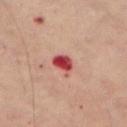No biopsy was performed on this lesion — it was imaged during a full skin examination and was not determined to be concerning. This image is a 15 mm lesion crop taken from a total-body photograph. The lesion-visualizer software estimated a footprint of about 4.5 mm², an outline eccentricity of about 0.7 (0 = round, 1 = elongated), and a shape-asymmetry score of about 0.3 (0 = symmetric). It also reported a normalized border contrast of about 12. The software also gave border irregularity of about 3 on a 0–10 scale, internal color variation of about 4 on a 0–10 scale, and a peripheral color-asymmetry measure near 1.5. A female patient, roughly 60 years of age. This is a cross-polarized tile. The lesion is on the left thigh.